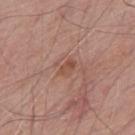follow-up: total-body-photography surveillance lesion; no biopsy
site: the back
acquisition: ~15 mm crop, total-body skin-cancer survey
subject: male, aged 63–67
size: ~2.5 mm (longest diameter)
lighting: white-light